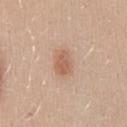Clinical impression:
No biopsy was performed on this lesion — it was imaged during a full skin examination and was not determined to be concerning.
Background:
Automated image analysis of the tile measured an area of roughly 5 mm², an eccentricity of roughly 0.75, and two-axis asymmetry of about 0.2. The analysis additionally found a border-irregularity rating of about 1.5/10, internal color variation of about 2.5 on a 0–10 scale, and peripheral color asymmetry of about 1. It also reported a nevus-likeness score of about 95/100 and a lesion-detection confidence of about 100/100. Cropped from a total-body skin-imaging series; the visible field is about 15 mm. The patient is a female aged approximately 30. Captured under white-light illumination. Longest diameter approximately 3 mm. From the mid back.